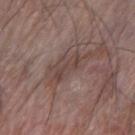{
  "biopsy_status": "not biopsied; imaged during a skin examination",
  "site": "arm",
  "lesion_size": {
    "long_diameter_mm_approx": 3.5
  },
  "patient": {
    "sex": "male",
    "age_approx": 65
  },
  "lighting": "white-light",
  "image": {
    "source": "total-body photography crop",
    "field_of_view_mm": 15
  }
}